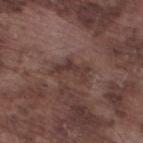notes — imaged on a skin check; not biopsied | lesion size — ≈4.5 mm | acquisition — ~15 mm crop, total-body skin-cancer survey | subject — male, aged around 75 | body site — the left lower leg | illumination — white-light.